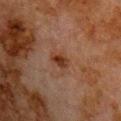Part of a total-body skin-imaging series; this lesion was reviewed on a skin check and was not flagged for biopsy. About 2.5 mm across. This is a cross-polarized tile. A close-up tile cropped from a whole-body skin photograph, about 15 mm across. The subject is a male about 80 years old. Automated tile analysis of the lesion measured a footprint of about 4.5 mm² and an eccentricity of roughly 0.65. On the front of the torso.